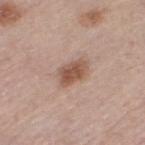Imaged during a routine full-body skin examination; the lesion was not biopsied and no histopathology is available.
Imaged with white-light lighting.
A lesion tile, about 15 mm wide, cut from a 3D total-body photograph.
The patient is a female roughly 65 years of age.
The lesion is located on the left thigh.
An algorithmic analysis of the crop reported an area of roughly 6.5 mm². The analysis additionally found a mean CIELAB color near L≈53 a*≈20 b*≈28, roughly 12 lightness units darker than nearby skin, and a normalized border contrast of about 8.5. The software also gave a border-irregularity index near 2/10, a within-lesion color-variation index near 3/10, and peripheral color asymmetry of about 1. The software also gave lesion-presence confidence of about 100/100.
Longest diameter approximately 3.5 mm.